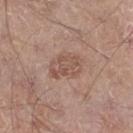Part of a total-body skin-imaging series; this lesion was reviewed on a skin check and was not flagged for biopsy. This image is a 15 mm lesion crop taken from a total-body photograph. From the leg. A male patient, aged approximately 60.